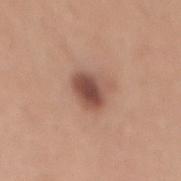Findings:
* follow-up: no biopsy performed (imaged during a skin exam)
* subject: female, in their mid- to late 30s
* anatomic site: the abdomen
* illumination: white-light illumination
* diameter: ≈3.5 mm
* acquisition: ~15 mm tile from a whole-body skin photo
* automated lesion analysis: a lesion area of about 8 mm² and an outline eccentricity of about 0.65 (0 = round, 1 = elongated); border irregularity of about 2.5 on a 0–10 scale, a within-lesion color-variation index near 4/10, and a peripheral color-asymmetry measure near 1